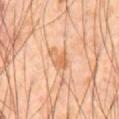follow-up=total-body-photography surveillance lesion; no biopsy | lesion diameter=~3 mm (longest diameter) | location=the abdomen | patient=male, approximately 65 years of age | acquisition=~15 mm tile from a whole-body skin photo.